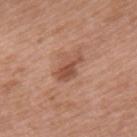Notes:
• biopsy status: no biopsy performed (imaged during a skin exam)
• patient: female, in their mid- to late 70s
• anatomic site: the upper back
• lighting: white-light
• image: 15 mm crop, total-body photography
• automated metrics: a symmetry-axis asymmetry near 0.3; border irregularity of about 3.5 on a 0–10 scale and peripheral color asymmetry of about 1